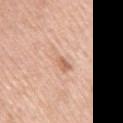Q: Is there a histopathology result?
A: total-body-photography surveillance lesion; no biopsy
Q: What did automated image analysis measure?
A: an area of roughly 6 mm², an outline eccentricity of about 0.95 (0 = round, 1 = elongated), and a shape-asymmetry score of about 0.4 (0 = symmetric); a lesion color around L≈67 a*≈20 b*≈32 in CIELAB and a lesion–skin lightness drop of about 8; an automated nevus-likeness rating near 0 out of 100 and a lesion-detection confidence of about 100/100
Q: What lighting was used for the tile?
A: white-light
Q: Lesion size?
A: about 4.5 mm
Q: What kind of image is this?
A: ~15 mm tile from a whole-body skin photo
Q: What are the patient's age and sex?
A: male, aged 53–57
Q: What is the anatomic site?
A: the left upper arm